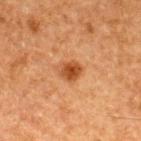follow-up = total-body-photography surveillance lesion; no biopsy
subject = male, aged 63–67
body site = the back
lesion diameter = ≈3 mm
illumination = cross-polarized illumination
imaging modality = 15 mm crop, total-body photography
automated metrics = a footprint of about 5 mm² and an eccentricity of roughly 0.55; a border-irregularity index near 2/10; a classifier nevus-likeness of about 95/100